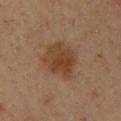The lesion is on the chest. The total-body-photography lesion software estimated two-axis asymmetry of about 0.2. A male patient, approximately 35 years of age. The lesion's longest dimension is about 5.5 mm. Imaged with cross-polarized lighting. Cropped from a whole-body photographic skin survey; the tile spans about 15 mm.